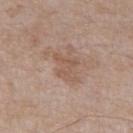notes: catalogued during a skin exam; not biopsied | lesion size: about 3.5 mm | site: the chest | acquisition: ~15 mm tile from a whole-body skin photo | subject: male, roughly 65 years of age | illumination: white-light.